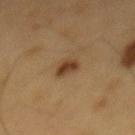biopsy status=total-body-photography surveillance lesion; no biopsy | image source=15 mm crop, total-body photography | location=the mid back | tile lighting=cross-polarized | size=≈3.5 mm | automated metrics=a lesion area of about 5 mm² and a shape eccentricity near 0.8; a within-lesion color-variation index near 3.5/10 and a peripheral color-asymmetry measure near 1.5 | patient=male, roughly 60 years of age.